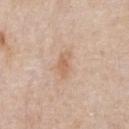workup — catalogued during a skin exam; not biopsied
automated lesion analysis — a footprint of about 4 mm², an outline eccentricity of about 0.9 (0 = round, 1 = elongated), and a shape-asymmetry score of about 0.35 (0 = symmetric); an average lesion color of about L≈63 a*≈19 b*≈32 (CIELAB), a lesion–skin lightness drop of about 8, and a normalized lesion–skin contrast near 6; a within-lesion color-variation index near 1.5/10 and radial color variation of about 0.5
imaging modality — 15 mm crop, total-body photography
lighting — white-light illumination
body site — the chest
patient — male, aged 58 to 62